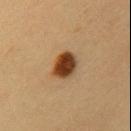No biopsy was performed on this lesion — it was imaged during a full skin examination and was not determined to be concerning. A 15 mm close-up tile from a total-body photography series done for melanoma screening. Automated tile analysis of the lesion measured a lesion color around L≈38 a*≈19 b*≈33 in CIELAB and roughly 17 lightness units darker than nearby skin. The software also gave a classifier nevus-likeness of about 100/100 and lesion-presence confidence of about 100/100. The patient is a female in their 30s. The tile uses cross-polarized illumination. On the left upper arm. Measured at roughly 3.5 mm in maximum diameter.